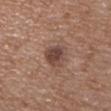The lesion was photographed on a routine skin check and not biopsied; there is no pathology result.
The patient is a female about 65 years old.
A 15 mm close-up extracted from a 3D total-body photography capture.
Automated tile analysis of the lesion measured an eccentricity of roughly 0.7 and two-axis asymmetry of about 0.2. The analysis additionally found a border-irregularity rating of about 2.5/10 and a peripheral color-asymmetry measure near 1. It also reported a classifier nevus-likeness of about 35/100 and a detector confidence of about 100 out of 100 that the crop contains a lesion.
Located on the upper back.
Imaged with white-light lighting.
Approximately 3.5 mm at its widest.